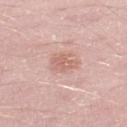biopsy status — catalogued during a skin exam; not biopsied
automated lesion analysis — an eccentricity of roughly 0.7 and a symmetry-axis asymmetry near 0.35; a mean CIELAB color near L≈63 a*≈22 b*≈26 and a normalized lesion–skin contrast near 5.5
patient — male, in their 50s
size — ≈3 mm
acquisition — ~15 mm tile from a whole-body skin photo
location — the left lower leg
tile lighting — white-light